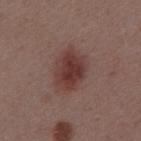This lesion was catalogued during total-body skin photography and was not selected for biopsy. The lesion is on the abdomen. A 15 mm close-up extracted from a 3D total-body photography capture. Automated tile analysis of the lesion measured an outline eccentricity of about 0.7 (0 = round, 1 = elongated). And it measured a lesion color around L≈37 a*≈22 b*≈21 in CIELAB, about 10 CIELAB-L* units darker than the surrounding skin, and a normalized lesion–skin contrast near 9. The analysis additionally found border irregularity of about 2 on a 0–10 scale, internal color variation of about 4 on a 0–10 scale, and peripheral color asymmetry of about 1. About 4.5 mm across. A male patient, about 55 years old. The tile uses white-light illumination.